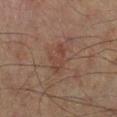The lesion is located on the leg.
The patient is a male in their mid-60s.
Cropped from a whole-body photographic skin survey; the tile spans about 15 mm.